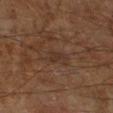workup: total-body-photography surveillance lesion; no biopsy
automated lesion analysis: an automated nevus-likeness rating near 0 out of 100
illumination: cross-polarized
imaging modality: ~15 mm tile from a whole-body skin photo
diameter: ~3 mm (longest diameter)
patient: male, in their 60s
anatomic site: the leg